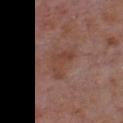No biopsy was performed on this lesion — it was imaged during a full skin examination and was not determined to be concerning.
Cropped from a whole-body photographic skin survey; the tile spans about 15 mm.
A male patient in their mid- to late 60s.
The lesion is located on the front of the torso.